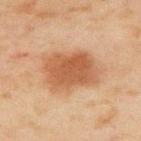Captured during whole-body skin photography for melanoma surveillance; the lesion was not biopsied.
On the right upper arm.
A close-up tile cropped from a whole-body skin photograph, about 15 mm across.
A male subject, roughly 45 years of age.